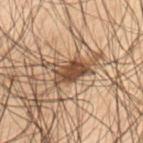Impression:
The lesion was tiled from a total-body skin photograph and was not biopsied.
Clinical summary:
Imaged with cross-polarized lighting. Automated tile analysis of the lesion measured an area of roughly 8 mm², an outline eccentricity of about 0.8 (0 = round, 1 = elongated), and two-axis asymmetry of about 0.3. It also reported a mean CIELAB color near L≈35 a*≈14 b*≈24 and a lesion–skin lightness drop of about 12. And it measured an automated nevus-likeness rating near 70 out of 100 and a lesion-detection confidence of about 90/100. On the left thigh. A close-up tile cropped from a whole-body skin photograph, about 15 mm across. The lesion's longest dimension is about 4 mm. A male patient, aged around 50.